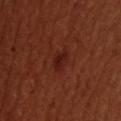Q: Was a biopsy performed?
A: total-body-photography surveillance lesion; no biopsy
Q: What is the imaging modality?
A: 15 mm crop, total-body photography
Q: Lesion location?
A: the upper back
Q: What are the patient's age and sex?
A: male, about 35 years old